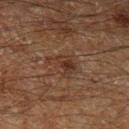• notes: imaged on a skin check; not biopsied